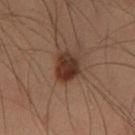{"biopsy_status": "not biopsied; imaged during a skin examination", "site": "left thigh", "automated_metrics": {"area_mm2_approx": 8.0, "shape_asymmetry": 0.2, "vs_skin_contrast_norm": 11.0, "border_irregularity_0_10": 2.0, "peripheral_color_asymmetry": 1.5, "nevus_likeness_0_100": 90, "lesion_detection_confidence_0_100": 100}, "patient": {"sex": "male", "age_approx": 55}, "image": {"source": "total-body photography crop", "field_of_view_mm": 15}}